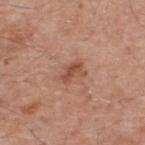notes: catalogued during a skin exam; not biopsied | location: the upper back | subject: male, aged 53–57 | tile lighting: white-light | image: ~15 mm tile from a whole-body skin photo.